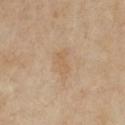The lesion was photographed on a routine skin check and not biopsied; there is no pathology result.
Approximately 4 mm at its widest.
The lesion is on the chest.
A female patient aged approximately 70.
A close-up tile cropped from a whole-body skin photograph, about 15 mm across.
The total-body-photography lesion software estimated a lesion area of about 6.5 mm². It also reported a lesion color around L≈62 a*≈15 b*≈34 in CIELAB. The analysis additionally found a border-irregularity index near 4.5/10 and internal color variation of about 1.5 on a 0–10 scale.
Imaged with cross-polarized lighting.